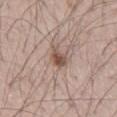Notes:
- image — total-body-photography crop, ~15 mm field of view
- tile lighting — white-light
- patient — male, roughly 70 years of age
- location — the right upper arm
- diameter — about 3.5 mm